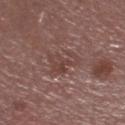| field | value |
|---|---|
| follow-up | total-body-photography surveillance lesion; no biopsy |
| image source | 15 mm crop, total-body photography |
| body site | the head or neck |
| size | ~4 mm (longest diameter) |
| tile lighting | white-light |
| patient | male, aged 53–57 |
| image-analysis metrics | an area of roughly 7.5 mm², an outline eccentricity of about 0.7 (0 = round, 1 = elongated), and a shape-asymmetry score of about 0.55 (0 = symmetric); a lesion color around L≈42 a*≈19 b*≈22 in CIELAB and a normalized border contrast of about 5.5 |